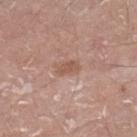{
  "biopsy_status": "not biopsied; imaged during a skin examination",
  "image": {
    "source": "total-body photography crop",
    "field_of_view_mm": 15
  },
  "lesion_size": {
    "long_diameter_mm_approx": 3.0
  },
  "site": "right lower leg",
  "lighting": "white-light",
  "patient": {
    "sex": "male",
    "age_approx": 80
  }
}